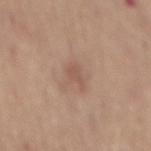Q: Was a biopsy performed?
A: no biopsy performed (imaged during a skin exam)
Q: How was the tile lit?
A: white-light illumination
Q: Lesion size?
A: about 2.5 mm
Q: What is the imaging modality?
A: ~15 mm tile from a whole-body skin photo
Q: Patient demographics?
A: male, roughly 50 years of age
Q: Automated lesion metrics?
A: a lesion–skin lightness drop of about 7; a border-irregularity index near 4/10, a color-variation rating of about 1/10, and peripheral color asymmetry of about 0.5; lesion-presence confidence of about 100/100
Q: What is the anatomic site?
A: the mid back